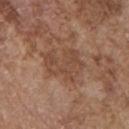notes: total-body-photography surveillance lesion; no biopsy
tile lighting: white-light illumination
patient: male, in their mid- to late 70s
acquisition: total-body-photography crop, ~15 mm field of view
location: the chest